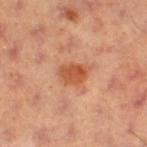notes: no biopsy performed (imaged during a skin exam); tile lighting: cross-polarized; body site: the leg; image source: total-body-photography crop, ~15 mm field of view; patient: female, aged 38–42.